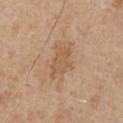Clinical impression:
Part of a total-body skin-imaging series; this lesion was reviewed on a skin check and was not flagged for biopsy.
Image and clinical context:
Located on the front of the torso. A lesion tile, about 15 mm wide, cut from a 3D total-body photograph. Approximately 4.5 mm at its widest. The patient is a male aged 63–67. Captured under white-light illumination.